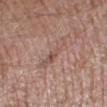notes = catalogued during a skin exam; not biopsied | site = the left lower leg | subject = male, aged approximately 85 or older | diameter = ~4.5 mm (longest diameter) | TBP lesion metrics = a mean CIELAB color near L≈53 a*≈19 b*≈24, about 6 CIELAB-L* units darker than the surrounding skin, and a normalized lesion–skin contrast near 4.5; a border-irregularity rating of about 5.5/10, internal color variation of about 5 on a 0–10 scale, and radial color variation of about 1.5; a lesion-detection confidence of about 100/100 | tile lighting = white-light | acquisition = ~15 mm crop, total-body skin-cancer survey.